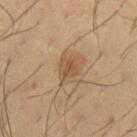biopsy_status: not biopsied; imaged during a skin examination
site: left upper arm
image:
  source: total-body photography crop
  field_of_view_mm: 15
patient:
  sex: male
  age_approx: 40